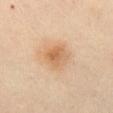follow-up = catalogued during a skin exam; not biopsied | image source = ~15 mm crop, total-body skin-cancer survey | body site = the left thigh | patient = female, about 45 years old.